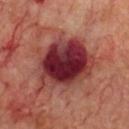Notes:
* workup · catalogued during a skin exam; not biopsied
* body site · the chest
* image · ~15 mm crop, total-body skin-cancer survey
* patient · male, approximately 70 years of age